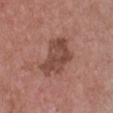<tbp_lesion>
<biopsy_status>not biopsied; imaged during a skin examination</biopsy_status>
<automated_metrics>
  <nevus_likeness_0_100>10</nevus_likeness_0_100>
  <lesion_detection_confidence_0_100>100</lesion_detection_confidence_0_100>
</automated_metrics>
<image>
  <source>total-body photography crop</source>
  <field_of_view_mm>15</field_of_view_mm>
</image>
<site>front of the torso</site>
<patient>
  <sex>female</sex>
  <age_approx>45</age_approx>
</patient>
<lesion_size>
  <long_diameter_mm_approx>5.0</long_diameter_mm_approx>
</lesion_size>
<lighting>white-light</lighting>
</tbp_lesion>